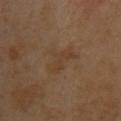Imaged during a routine full-body skin examination; the lesion was not biopsied and no histopathology is available. The lesion is located on the upper back. A female patient, in their 40s. The recorded lesion diameter is about 5 mm. This is a cross-polarized tile. This image is a 15 mm lesion crop taken from a total-body photograph. Automated image analysis of the tile measured roughly 4 lightness units darker than nearby skin.